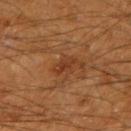notes=no biopsy performed (imaged during a skin exam); acquisition=total-body-photography crop, ~15 mm field of view; diameter=~3 mm (longest diameter); anatomic site=the right upper arm; patient=male, aged approximately 60; tile lighting=cross-polarized.